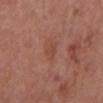Recorded during total-body skin imaging; not selected for excision or biopsy. Cropped from a whole-body photographic skin survey; the tile spans about 15 mm. The patient is a male aged around 55. This is a white-light tile. On the chest. The lesion-visualizer software estimated a mean CIELAB color near L≈46 a*≈23 b*≈30 and a lesion-to-skin contrast of about 5 (normalized; higher = more distinct). And it measured a border-irregularity rating of about 4/10 and a peripheral color-asymmetry measure near 0.5. Measured at roughly 2.5 mm in maximum diameter.